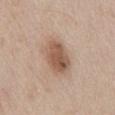workup: total-body-photography surveillance lesion; no biopsy | lighting: white-light | patient: male, aged around 65 | body site: the abdomen | acquisition: 15 mm crop, total-body photography | diameter: ≈4.5 mm.